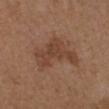A female subject about 40 years old. The lesion-visualizer software estimated a footprint of about 15 mm², a shape eccentricity near 0.85, and two-axis asymmetry of about 0.55. The software also gave a border-irregularity rating of about 6.5/10, a within-lesion color-variation index near 3/10, and radial color variation of about 1. Longest diameter approximately 6 mm. On the left forearm. The tile uses white-light illumination. A 15 mm close-up extracted from a 3D total-body photography capture.